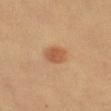– follow-up · no biopsy performed (imaged during a skin exam)
– imaging modality · 15 mm crop, total-body photography
– tile lighting · cross-polarized illumination
– automated metrics · a color-variation rating of about 3/10 and peripheral color asymmetry of about 1; a classifier nevus-likeness of about 100/100 and lesion-presence confidence of about 100/100
– patient · aged 58 to 62
– lesion diameter · ~3 mm (longest diameter)
– body site · the left thigh